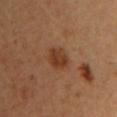The lesion was photographed on a routine skin check and not biopsied; there is no pathology result. On the left upper arm. A 15 mm crop from a total-body photograph taken for skin-cancer surveillance. About 3 mm across. An algorithmic analysis of the crop reported a lesion area of about 6 mm², an outline eccentricity of about 0.65 (0 = round, 1 = elongated), and a symmetry-axis asymmetry near 0.15. The software also gave a lesion color around L≈38 a*≈22 b*≈33 in CIELAB, about 9 CIELAB-L* units darker than the surrounding skin, and a normalized lesion–skin contrast near 8. And it measured an automated nevus-likeness rating near 85 out of 100 and lesion-presence confidence of about 100/100. Captured under cross-polarized illumination. A female patient, roughly 35 years of age.